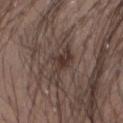<record>
<biopsy_status>not biopsied; imaged during a skin examination</biopsy_status>
<patient>
  <sex>male</sex>
  <age_approx>35</age_approx>
</patient>
<site>arm</site>
<image>
  <source>total-body photography crop</source>
  <field_of_view_mm>15</field_of_view_mm>
</image>
<lighting>white-light</lighting>
</record>